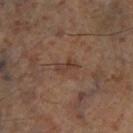Captured during whole-body skin photography for melanoma surveillance; the lesion was not biopsied.